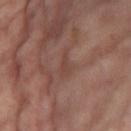{
  "biopsy_status": "not biopsied; imaged during a skin examination",
  "site": "left thigh",
  "image": {
    "source": "total-body photography crop",
    "field_of_view_mm": 15
  },
  "patient": {
    "sex": "female",
    "age_approx": 55
  }
}